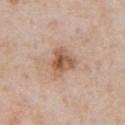Q: Is there a histopathology result?
A: total-body-photography surveillance lesion; no biopsy
Q: How large is the lesion?
A: about 4 mm
Q: Illumination type?
A: white-light
Q: Lesion location?
A: the chest
Q: Automated lesion metrics?
A: a nevus-likeness score of about 35/100 and a lesion-detection confidence of about 100/100
Q: Who is the patient?
A: male, aged around 65
Q: How was this image acquired?
A: ~15 mm crop, total-body skin-cancer survey